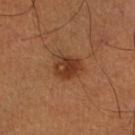Assessment:
This lesion was catalogued during total-body skin photography and was not selected for biopsy.
Acquisition and patient details:
A male subject, aged approximately 50. The lesion's longest dimension is about 3.5 mm. Cropped from a whole-body photographic skin survey; the tile spans about 15 mm. Captured under cross-polarized illumination. The lesion is located on the right lower leg.